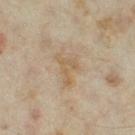workup = imaged on a skin check; not biopsied
image-analysis metrics = an area of roughly 5.5 mm², an eccentricity of roughly 0.8, and a symmetry-axis asymmetry near 0.65
diameter = about 4 mm
location = the leg
patient = female, aged around 35
tile lighting = cross-polarized
imaging modality = ~15 mm crop, total-body skin-cancer survey